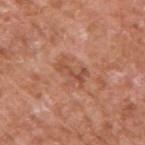Context:
A region of skin cropped from a whole-body photographic capture, roughly 15 mm wide. The patient is a male approximately 65 years of age. The lesion is located on the right upper arm.